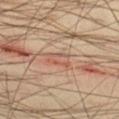Impression: Part of a total-body skin-imaging series; this lesion was reviewed on a skin check and was not flagged for biopsy. Acquisition and patient details: From the right thigh. This image is a 15 mm lesion crop taken from a total-body photograph. Measured at roughly 3 mm in maximum diameter. This is a cross-polarized tile. A male patient, about 40 years old.